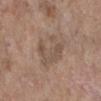{"biopsy_status": "not biopsied; imaged during a skin examination", "patient": {"sex": "female", "age_approx": "85+"}, "site": "right lower leg", "image": {"source": "total-body photography crop", "field_of_view_mm": 15}}